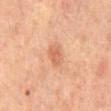Recorded during total-body skin imaging; not selected for excision or biopsy. A close-up tile cropped from a whole-body skin photograph, about 15 mm across. A male subject, in their mid- to late 60s. The lesion is located on the mid back.